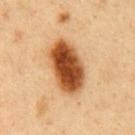Impression:
This lesion was catalogued during total-body skin photography and was not selected for biopsy.
Background:
Cropped from a whole-body photographic skin survey; the tile spans about 15 mm. A male patient, roughly 50 years of age. From the chest.